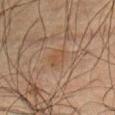Imaged during a routine full-body skin examination; the lesion was not biopsied and no histopathology is available. The total-body-photography lesion software estimated a mean CIELAB color near L≈41 a*≈15 b*≈28, a lesion–skin lightness drop of about 4, and a normalized lesion–skin contrast near 5. Cropped from a total-body skin-imaging series; the visible field is about 15 mm. On the abdomen. Approximately 3 mm at its widest. The patient is a male approximately 60 years of age. This is a cross-polarized tile.